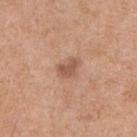{"site": "chest", "image": {"source": "total-body photography crop", "field_of_view_mm": 15}, "patient": {"sex": "male", "age_approx": 55}, "lighting": "white-light", "lesion_size": {"long_diameter_mm_approx": 3.0}}